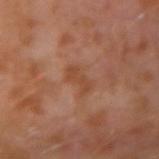biopsy status = catalogued during a skin exam; not biopsied
image = ~15 mm tile from a whole-body skin photo
lighting = cross-polarized illumination
subject = male, aged around 30
automated lesion analysis = a lesion area of about 4 mm²; a border-irregularity index near 4/10, internal color variation of about 1.5 on a 0–10 scale, and a peripheral color-asymmetry measure near 0.5; a classifier nevus-likeness of about 0/100 and a lesion-detection confidence of about 100/100
lesion diameter = ~3 mm (longest diameter)
site = the left arm